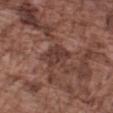Clinical summary:
Located on the arm. A male patient, aged 73 to 77. This image is a 15 mm lesion crop taken from a total-body photograph.